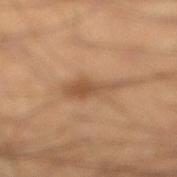Imaged during a routine full-body skin examination; the lesion was not biopsied and no histopathology is available.
Cropped from a total-body skin-imaging series; the visible field is about 15 mm.
Automated image analysis of the tile measured a mean CIELAB color near L≈52 a*≈19 b*≈34, about 10 CIELAB-L* units darker than the surrounding skin, and a lesion-to-skin contrast of about 7 (normalized; higher = more distinct). And it measured an automated nevus-likeness rating near 5 out of 100 and lesion-presence confidence of about 100/100.
A male patient, aged approximately 40.
The lesion is located on the right lower leg.
The recorded lesion diameter is about 5 mm.